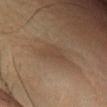Q: Was a biopsy performed?
A: catalogued during a skin exam; not biopsied
Q: What is the imaging modality?
A: ~15 mm tile from a whole-body skin photo
Q: What is the anatomic site?
A: the leg
Q: What is the lesion's diameter?
A: about 4.5 mm
Q: Who is the patient?
A: male, aged 58–62